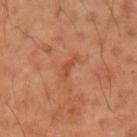Captured during whole-body skin photography for melanoma surveillance; the lesion was not biopsied.
Automated tile analysis of the lesion measured a lesion area of about 3.5 mm², an eccentricity of roughly 0.95, and a symmetry-axis asymmetry near 0.5. And it measured a mean CIELAB color near L≈50 a*≈27 b*≈35, a lesion–skin lightness drop of about 7, and a normalized lesion–skin contrast near 5.5. The analysis additionally found a border-irregularity index near 5.5/10, internal color variation of about 0 on a 0–10 scale, and a peripheral color-asymmetry measure near 0.
The lesion is located on the left upper arm.
About 3.5 mm across.
The tile uses cross-polarized illumination.
A male subject aged around 45.
A 15 mm crop from a total-body photograph taken for skin-cancer surveillance.